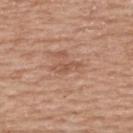Q: Was this lesion biopsied?
A: no biopsy performed (imaged during a skin exam)
Q: What is the lesion's diameter?
A: about 3 mm
Q: What kind of image is this?
A: ~15 mm crop, total-body skin-cancer survey
Q: What is the anatomic site?
A: the upper back
Q: Patient demographics?
A: female, roughly 60 years of age
Q: Automated lesion metrics?
A: an average lesion color of about L≈54 a*≈22 b*≈30 (CIELAB), a lesion–skin lightness drop of about 8, and a normalized border contrast of about 5.5; peripheral color asymmetry of about 0
Q: How was the tile lit?
A: white-light illumination